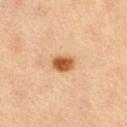<record>
<biopsy_status>not biopsied; imaged during a skin examination</biopsy_status>
<lesion_size>
  <long_diameter_mm_approx>2.5</long_diameter_mm_approx>
</lesion_size>
<site>right thigh</site>
<patient>
  <sex>female</sex>
  <age_approx>65</age_approx>
</patient>
<image>
  <source>total-body photography crop</source>
  <field_of_view_mm>15</field_of_view_mm>
</image>
</record>